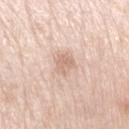Captured during whole-body skin photography for melanoma surveillance; the lesion was not biopsied. A lesion tile, about 15 mm wide, cut from a 3D total-body photograph. The lesion is located on the left upper arm. The lesion's longest dimension is about 3 mm. Automated image analysis of the tile measured a border-irregularity index near 3/10 and radial color variation of about 1. It also reported a nevus-likeness score of about 0/100. The patient is a female about 65 years old.